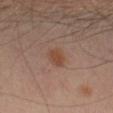Findings:
- notes: imaged on a skin check; not biopsied
- acquisition: ~15 mm crop, total-body skin-cancer survey
- subject: male, aged around 65
- illumination: cross-polarized illumination
- lesion size: ~3 mm (longest diameter)
- location: the left thigh